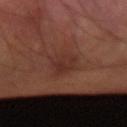Q: Was a biopsy performed?
A: catalogued during a skin exam; not biopsied
Q: What is the imaging modality?
A: 15 mm crop, total-body photography
Q: Who is the patient?
A: male, in their 60s
Q: Where on the body is the lesion?
A: the right arm
Q: What lighting was used for the tile?
A: cross-polarized illumination